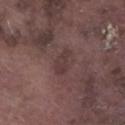Part of a total-body skin-imaging series; this lesion was reviewed on a skin check and was not flagged for biopsy.
The tile uses white-light illumination.
The lesion is on the left lower leg.
An algorithmic analysis of the crop reported a lesion area of about 4.5 mm², an outline eccentricity of about 0.8 (0 = round, 1 = elongated), and a shape-asymmetry score of about 0.35 (0 = symmetric). It also reported a lesion color around L≈36 a*≈17 b*≈17 in CIELAB and roughly 6 lightness units darker than nearby skin. It also reported border irregularity of about 3.5 on a 0–10 scale, internal color variation of about 1.5 on a 0–10 scale, and a peripheral color-asymmetry measure near 0.5.
The subject is a male aged 73 to 77.
This image is a 15 mm lesion crop taken from a total-body photograph.